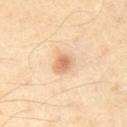Captured during whole-body skin photography for melanoma surveillance; the lesion was not biopsied.
Automated tile analysis of the lesion measured an eccentricity of roughly 0.6 and a shape-asymmetry score of about 0.15 (0 = symmetric). The analysis additionally found a mean CIELAB color near L≈68 a*≈21 b*≈36, roughly 12 lightness units darker than nearby skin, and a normalized border contrast of about 7. The analysis additionally found a lesion-detection confidence of about 100/100.
A male subject aged approximately 50.
A 15 mm close-up extracted from a 3D total-body photography capture.
The tile uses cross-polarized illumination.
The lesion is located on the chest.